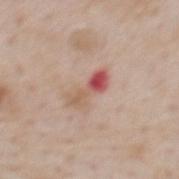Part of a total-body skin-imaging series; this lesion was reviewed on a skin check and was not flagged for biopsy. A male patient in their mid- to late 60s. From the back. Automated image analysis of the tile measured a mean CIELAB color near L≈57 a*≈24 b*≈27, a lesion–skin lightness drop of about 11, and a normalized lesion–skin contrast near 7.5. The analysis additionally found a border-irregularity index near 5/10, a within-lesion color-variation index near 10/10, and radial color variation of about 8. This image is a 15 mm lesion crop taken from a total-body photograph.